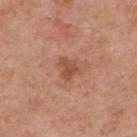follow-up: total-body-photography surveillance lesion; no biopsy | automated lesion analysis: a footprint of about 4.5 mm² and a symmetry-axis asymmetry near 0.45; a lesion color around L≈51 a*≈25 b*≈33 in CIELAB and about 9 CIELAB-L* units darker than the surrounding skin; a border-irregularity index near 4.5/10, internal color variation of about 2 on a 0–10 scale, and peripheral color asymmetry of about 0.5; an automated nevus-likeness rating near 10 out of 100 and lesion-presence confidence of about 100/100 | patient: male, roughly 55 years of age | size: ~3 mm (longest diameter) | acquisition: total-body-photography crop, ~15 mm field of view | location: the upper back | lighting: white-light.